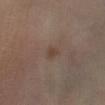Captured during whole-body skin photography for melanoma surveillance; the lesion was not biopsied. Automated tile analysis of the lesion measured border irregularity of about 1.5 on a 0–10 scale. This is a cross-polarized tile. A 15 mm close-up tile from a total-body photography series done for melanoma screening. The lesion is located on the left lower leg. The recorded lesion diameter is about 1.5 mm. A male patient approximately 45 years of age.